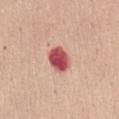Clinical impression: Recorded during total-body skin imaging; not selected for excision or biopsy. Clinical summary: Automated image analysis of the tile measured an area of roughly 7.5 mm² and an outline eccentricity of about 0.7 (0 = round, 1 = elongated). The analysis additionally found a mean CIELAB color near L≈53 a*≈35 b*≈24, roughly 19 lightness units darker than nearby skin, and a normalized border contrast of about 12. The subject is a female aged around 50. The lesion is on the abdomen. A lesion tile, about 15 mm wide, cut from a 3D total-body photograph. Imaged with white-light lighting. Approximately 3.5 mm at its widest.